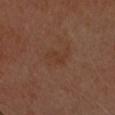Impression:
The lesion was tiled from a total-body skin photograph and was not biopsied.
Acquisition and patient details:
An algorithmic analysis of the crop reported an outline eccentricity of about 0.8 (0 = round, 1 = elongated) and a shape-asymmetry score of about 0.45 (0 = symmetric). The lesion's longest dimension is about 3.5 mm. A 15 mm close-up tile from a total-body photography series done for melanoma screening. A female subject. This is a cross-polarized tile. The lesion is located on the head or neck.